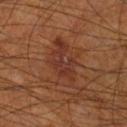The lesion was tiled from a total-body skin photograph and was not biopsied. Approximately 6 mm at its widest. The lesion is on the right lower leg. This image is a 15 mm lesion crop taken from a total-body photograph. An algorithmic analysis of the crop reported a lesion area of about 15 mm² and two-axis asymmetry of about 0.25. And it measured a border-irregularity index near 4/10, a within-lesion color-variation index near 4.5/10, and radial color variation of about 1.5. It also reported a classifier nevus-likeness of about 0/100 and a lesion-detection confidence of about 100/100. The patient is a male aged around 70.